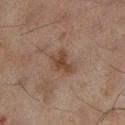<lesion>
<biopsy_status>not biopsied; imaged during a skin examination</biopsy_status>
<lighting>cross-polarized</lighting>
<patient>
  <sex>male</sex>
  <age_approx>45</age_approx>
</patient>
<site>left lower leg</site>
<image>
  <source>total-body photography crop</source>
  <field_of_view_mm>15</field_of_view_mm>
</image>
<lesion_size>
  <long_diameter_mm_approx>3.0</long_diameter_mm_approx>
</lesion_size>
<automated_metrics>
  <cielab_L>34</cielab_L>
  <cielab_a>15</cielab_a>
  <cielab_b>23</cielab_b>
  <vs_skin_darker_L>7.0</vs_skin_darker_L>
  <vs_skin_contrast_norm>7.5</vs_skin_contrast_norm>
</automated_metrics>
</lesion>